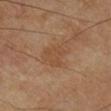Case summary:
- patient — male, in their mid-60s
- site — the leg
- imaging modality — total-body-photography crop, ~15 mm field of view
- lesion size — about 4 mm